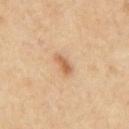  biopsy_status: not biopsied; imaged during a skin examination
  site: mid back
  image:
    source: total-body photography crop
    field_of_view_mm: 15
  patient:
    sex: male
    age_approx: 55